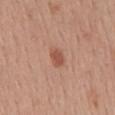Q: Illumination type?
A: white-light illumination
Q: What is the imaging modality?
A: ~15 mm crop, total-body skin-cancer survey
Q: Where on the body is the lesion?
A: the mid back
Q: How large is the lesion?
A: ≈2.5 mm
Q: Who is the patient?
A: female, approximately 55 years of age